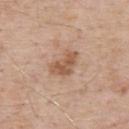Captured during whole-body skin photography for melanoma surveillance; the lesion was not biopsied. From the back. A male patient roughly 60 years of age. The tile uses white-light illumination. Approximately 4 mm at its widest. The lesion-visualizer software estimated an outline eccentricity of about 0.85 (0 = round, 1 = elongated) and a shape-asymmetry score of about 0.35 (0 = symmetric). The software also gave an automated nevus-likeness rating near 20 out of 100 and a lesion-detection confidence of about 100/100. A 15 mm close-up extracted from a 3D total-body photography capture.